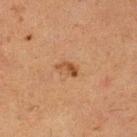biopsy_status: not biopsied; imaged during a skin examination
site: left lower leg
lighting: cross-polarized
lesion_size:
  long_diameter_mm_approx: 3.0
image:
  source: total-body photography crop
  field_of_view_mm: 15
automated_metrics:
  area_mm2_approx: 3.5
  eccentricity: 0.85
  border_irregularity_0_10: 3.5
patient:
  sex: female
  age_approx: 50